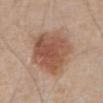acquisition = ~15 mm tile from a whole-body skin photo; site = the abdomen; patient = male, aged 78 to 82.